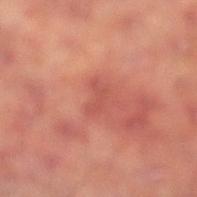Captured during whole-body skin photography for melanoma surveillance; the lesion was not biopsied.
A region of skin cropped from a whole-body photographic capture, roughly 15 mm wide.
A male patient roughly 70 years of age.
This is a cross-polarized tile.
Located on the leg.
The recorded lesion diameter is about 3 mm.